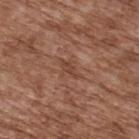This image is a 15 mm lesion crop taken from a total-body photograph.
On the upper back.
The recorded lesion diameter is about 3 mm.
Automated tile analysis of the lesion measured border irregularity of about 3 on a 0–10 scale and a within-lesion color-variation index near 0/10.
The patient is a male in their mid-70s.
This is a white-light tile.